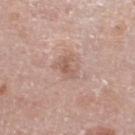Clinical impression:
Recorded during total-body skin imaging; not selected for excision or biopsy.
Clinical summary:
Longest diameter approximately 3 mm. Captured under white-light illumination. A lesion tile, about 15 mm wide, cut from a 3D total-body photograph. Located on the leg. The patient is a male roughly 80 years of age.